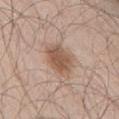The lesion was tiled from a total-body skin photograph and was not biopsied.
Located on the lower back.
Imaged with white-light lighting.
Cropped from a total-body skin-imaging series; the visible field is about 15 mm.
The total-body-photography lesion software estimated a lesion area of about 9.5 mm², an eccentricity of roughly 0.65, and two-axis asymmetry of about 0.15. The analysis additionally found border irregularity of about 2 on a 0–10 scale, internal color variation of about 3.5 on a 0–10 scale, and peripheral color asymmetry of about 1. The software also gave a nevus-likeness score of about 95/100 and a lesion-detection confidence of about 100/100.
Measured at roughly 4 mm in maximum diameter.
A male patient, aged around 70.